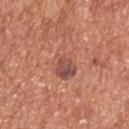The lesion was tiled from a total-body skin photograph and was not biopsied.
Cropped from a whole-body photographic skin survey; the tile spans about 15 mm.
A male subject approximately 65 years of age.
The lesion is on the upper back.